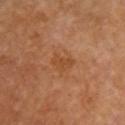workup = total-body-photography surveillance lesion; no biopsy | image source = ~15 mm tile from a whole-body skin photo | location = the chest | patient = female, aged 63 to 67.